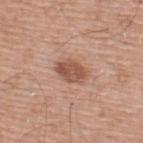Clinical impression:
Recorded during total-body skin imaging; not selected for excision or biopsy.
Context:
From the back. A roughly 15 mm field-of-view crop from a total-body skin photograph. The lesion-visualizer software estimated an average lesion color of about L≈53 a*≈22 b*≈29 (CIELAB), roughly 12 lightness units darker than nearby skin, and a normalized border contrast of about 8. The analysis additionally found a nevus-likeness score of about 70/100 and a lesion-detection confidence of about 100/100. A male subject, approximately 55 years of age.